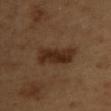workup = no biopsy performed (imaged during a skin exam); patient = female, approximately 40 years of age; site = the upper back; image source = total-body-photography crop, ~15 mm field of view.